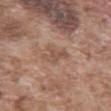notes: no biopsy performed (imaged during a skin exam) | illumination: white-light illumination | subject: male, approximately 75 years of age | image-analysis metrics: an eccentricity of roughly 0.85 and two-axis asymmetry of about 0.45; an average lesion color of about L≈51 a*≈19 b*≈27 (CIELAB) and a lesion-to-skin contrast of about 5.5 (normalized; higher = more distinct); a border-irregularity rating of about 5.5/10 and a color-variation rating of about 1.5/10 | location: the front of the torso | image: total-body-photography crop, ~15 mm field of view.